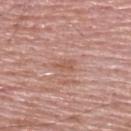{"biopsy_status": "not biopsied; imaged during a skin examination", "site": "upper back", "patient": {"sex": "male", "age_approx": 65}, "image": {"source": "total-body photography crop", "field_of_view_mm": 15}}